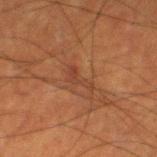The lesion was tiled from a total-body skin photograph and was not biopsied.
The tile uses cross-polarized illumination.
Cropped from a total-body skin-imaging series; the visible field is about 15 mm.
About 3 mm across.
From the leg.
An algorithmic analysis of the crop reported a shape eccentricity near 0.9. The software also gave an average lesion color of about L≈32 a*≈20 b*≈26 (CIELAB), roughly 5 lightness units darker than nearby skin, and a normalized lesion–skin contrast near 5.5. It also reported a border-irregularity index near 7.5/10, a color-variation rating of about 0/10, and peripheral color asymmetry of about 0. And it measured a classifier nevus-likeness of about 0/100 and a lesion-detection confidence of about 85/100.
A male patient aged around 50.